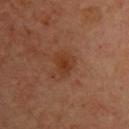The lesion was tiled from a total-body skin photograph and was not biopsied. A male patient approximately 65 years of age. An algorithmic analysis of the crop reported a mean CIELAB color near L≈36 a*≈23 b*≈32, a lesion–skin lightness drop of about 7, and a lesion-to-skin contrast of about 7 (normalized; higher = more distinct). This is a cross-polarized tile. The recorded lesion diameter is about 3 mm. A 15 mm close-up extracted from a 3D total-body photography capture. On the upper back.